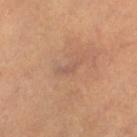This lesion was catalogued during total-body skin photography and was not selected for biopsy.
The lesion is on the leg.
A close-up tile cropped from a whole-body skin photograph, about 15 mm across.
The patient is a female aged 28 to 32.
The tile uses cross-polarized illumination.